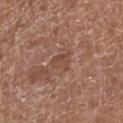This lesion was catalogued during total-body skin photography and was not selected for biopsy.
On the leg.
Cropped from a total-body skin-imaging series; the visible field is about 15 mm.
Longest diameter approximately 2.5 mm.
A male subject, aged around 75.